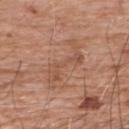Q: Was a biopsy performed?
A: imaged on a skin check; not biopsied